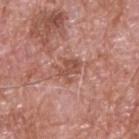On the upper back.
The subject is a male aged around 60.
Measured at roughly 2.5 mm in maximum diameter.
A roughly 15 mm field-of-view crop from a total-body skin photograph.